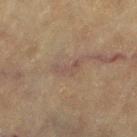Q: Was a biopsy performed?
A: total-body-photography surveillance lesion; no biopsy
Q: Lesion size?
A: about 3 mm
Q: What kind of image is this?
A: ~15 mm crop, total-body skin-cancer survey
Q: Lesion location?
A: the left thigh
Q: How was the tile lit?
A: cross-polarized
Q: What are the patient's age and sex?
A: female, aged around 80
Q: What did automated image analysis measure?
A: a border-irregularity rating of about 6.5/10, internal color variation of about 0 on a 0–10 scale, and peripheral color asymmetry of about 0